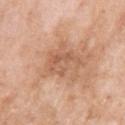{"biopsy_status": "not biopsied; imaged during a skin examination", "site": "left upper arm", "lighting": "white-light", "patient": {"sex": "female", "age_approx": 70}, "image": {"source": "total-body photography crop", "field_of_view_mm": 15}}